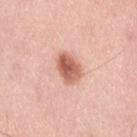Case summary:
* notes — total-body-photography surveillance lesion; no biopsy
* lesion size — about 3.5 mm
* illumination — white-light illumination
* subject — female, aged approximately 50
* TBP lesion metrics — an average lesion color of about L≈61 a*≈26 b*≈31 (CIELAB), a lesion–skin lightness drop of about 15, and a normalized border contrast of about 9.5; border irregularity of about 2 on a 0–10 scale and a peripheral color-asymmetry measure near 2
* site — the lower back
* image — 15 mm crop, total-body photography